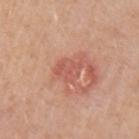The lesion was tiled from a total-body skin photograph and was not biopsied.
The total-body-photography lesion software estimated a footprint of about 6 mm², a shape eccentricity near 0.6, and a shape-asymmetry score of about 0.3 (0 = symmetric). It also reported a lesion color around L≈57 a*≈28 b*≈29 in CIELAB and about 8 CIELAB-L* units darker than the surrounding skin. It also reported an automated nevus-likeness rating near 0 out of 100.
A 15 mm close-up extracted from a 3D total-body photography capture.
The lesion is located on the left upper arm.
A female patient about 45 years old.
The recorded lesion diameter is about 3.5 mm.